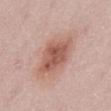| field | value |
|---|---|
| workup | total-body-photography surveillance lesion; no biopsy |
| anatomic site | the mid back |
| illumination | white-light |
| TBP lesion metrics | an area of roughly 14 mm², an eccentricity of roughly 0.65, and a shape-asymmetry score of about 0.15 (0 = symmetric); a mean CIELAB color near L≈56 a*≈24 b*≈27, roughly 11 lightness units darker than nearby skin, and a normalized lesion–skin contrast near 8; border irregularity of about 1.5 on a 0–10 scale, internal color variation of about 4 on a 0–10 scale, and a peripheral color-asymmetry measure near 1.5; a nevus-likeness score of about 85/100 and a detector confidence of about 100 out of 100 that the crop contains a lesion |
| acquisition | ~15 mm crop, total-body skin-cancer survey |
| patient | female, aged 48 to 52 |
| lesion size | about 4.5 mm |